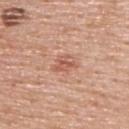{"biopsy_status": "not biopsied; imaged during a skin examination", "image": {"source": "total-body photography crop", "field_of_view_mm": 15}, "lighting": "white-light", "site": "upper back", "lesion_size": {"long_diameter_mm_approx": 2.5}, "patient": {"sex": "male", "age_approx": 60}}